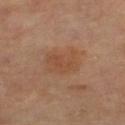workup: no biopsy performed (imaged during a skin exam); location: the leg; illumination: cross-polarized illumination; image: 15 mm crop, total-body photography; patient: female, roughly 70 years of age; size: ≈4.5 mm.